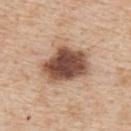Clinical impression: The lesion was tiled from a total-body skin photograph and was not biopsied. Acquisition and patient details: Automated tile analysis of the lesion measured border irregularity of about 2.5 on a 0–10 scale and a within-lesion color-variation index near 6/10. The analysis additionally found a nevus-likeness score of about 80/100 and a lesion-detection confidence of about 100/100. Longest diameter approximately 6 mm. The lesion is located on the upper back. A male patient aged approximately 60. Cropped from a whole-body photographic skin survey; the tile spans about 15 mm. Imaged with white-light lighting.